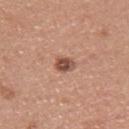{
  "biopsy_status": "not biopsied; imaged during a skin examination",
  "lighting": "white-light",
  "automated_metrics": {
    "eccentricity": 0.7,
    "shape_asymmetry": 0.2,
    "cielab_L": 50,
    "cielab_a": 22,
    "cielab_b": 28,
    "vs_skin_darker_L": 14.0,
    "vs_skin_contrast_norm": 9.5,
    "border_irregularity_0_10": 2.0,
    "color_variation_0_10": 4.5,
    "peripheral_color_asymmetry": 1.5
  },
  "site": "left upper arm",
  "patient": {
    "sex": "male",
    "age_approx": 40
  },
  "image": {
    "source": "total-body photography crop",
    "field_of_view_mm": 15
  },
  "lesion_size": {
    "long_diameter_mm_approx": 2.5
  }
}